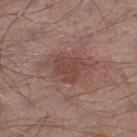Impression:
The lesion was photographed on a routine skin check and not biopsied; there is no pathology result.
Clinical summary:
The subject is a male aged approximately 55. This is a white-light tile. A region of skin cropped from a whole-body photographic capture, roughly 15 mm wide. The lesion is located on the right lower leg. About 3.5 mm across. An algorithmic analysis of the crop reported a lesion area of about 8.5 mm² and a shape eccentricity near 0.5. The software also gave roughly 8 lightness units darker than nearby skin and a normalized border contrast of about 6.5. The analysis additionally found a nevus-likeness score of about 0/100 and lesion-presence confidence of about 100/100.